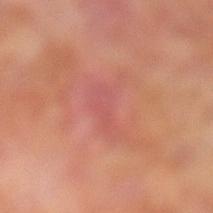Imaged during a routine full-body skin examination; the lesion was not biopsied and no histopathology is available. This is a cross-polarized tile. The lesion is on the left lower leg. A close-up tile cropped from a whole-body skin photograph, about 15 mm across. Automated tile analysis of the lesion measured a normalized lesion–skin contrast near 4.5. The software also gave a border-irregularity index near 7/10 and a within-lesion color-variation index near 3/10. The subject is a male roughly 70 years of age. Longest diameter approximately 5 mm.